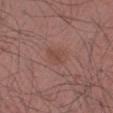Q: Was a biopsy performed?
A: no biopsy performed (imaged during a skin exam)
Q: What kind of image is this?
A: ~15 mm tile from a whole-body skin photo
Q: What is the lesion's diameter?
A: ≈3 mm
Q: Who is the patient?
A: male, aged approximately 40
Q: Automated lesion metrics?
A: a lesion area of about 4.5 mm² and a shape-asymmetry score of about 0.15 (0 = symmetric); border irregularity of about 1.5 on a 0–10 scale and peripheral color asymmetry of about 0.5; an automated nevus-likeness rating near 10 out of 100
Q: Where on the body is the lesion?
A: the right upper arm
Q: How was the tile lit?
A: white-light illumination